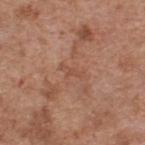Q: Was a biopsy performed?
A: total-body-photography surveillance lesion; no biopsy
Q: What are the patient's age and sex?
A: male, roughly 60 years of age
Q: What kind of image is this?
A: ~15 mm crop, total-body skin-cancer survey
Q: Lesion location?
A: the back